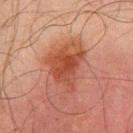Assessment:
Captured during whole-body skin photography for melanoma surveillance; the lesion was not biopsied.
Acquisition and patient details:
On the chest. Cropped from a total-body skin-imaging series; the visible field is about 15 mm. A male patient, aged approximately 45. The tile uses cross-polarized illumination. The lesion's longest dimension is about 5 mm. Automated image analysis of the tile measured a lesion color around L≈39 a*≈25 b*≈29 in CIELAB and a lesion-to-skin contrast of about 8.5 (normalized; higher = more distinct). It also reported border irregularity of about 5 on a 0–10 scale, a within-lesion color-variation index near 4/10, and a peripheral color-asymmetry measure near 1.